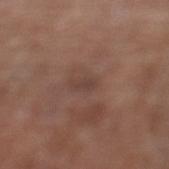workup — total-body-photography surveillance lesion; no biopsy | body site — the left lower leg | image — ~15 mm tile from a whole-body skin photo | subject — female, aged 78–82 | tile lighting — white-light | automated lesion analysis — a lesion area of about 2.5 mm², an eccentricity of roughly 0.95, and a symmetry-axis asymmetry near 0.25; roughly 6 lightness units darker than nearby skin and a lesion-to-skin contrast of about 5.5 (normalized; higher = more distinct); internal color variation of about 0 on a 0–10 scale | diameter — about 3 mm.